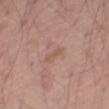{
  "biopsy_status": "not biopsied; imaged during a skin examination",
  "patient": {
    "sex": "male",
    "age_approx": 55
  },
  "site": "right thigh",
  "automated_metrics": {
    "area_mm2_approx": 3.0,
    "eccentricity": 0.95,
    "shape_asymmetry": 0.35,
    "cielab_L": 55,
    "cielab_a": 20,
    "cielab_b": 27,
    "vs_skin_darker_L": 6.0,
    "vs_skin_contrast_norm": 5.0,
    "border_irregularity_0_10": 3.5,
    "color_variation_0_10": 0.0,
    "peripheral_color_asymmetry": 0.0,
    "nevus_likeness_0_100": 0,
    "lesion_detection_confidence_0_100": 100
  },
  "image": {
    "source": "total-body photography crop",
    "field_of_view_mm": 15
  },
  "lesion_size": {
    "long_diameter_mm_approx": 3.0
  }
}